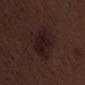Clinical impression: This lesion was catalogued during total-body skin photography and was not selected for biopsy. Image and clinical context: Measured at roughly 6 mm in maximum diameter. This is a white-light tile. Located on the left thigh. The subject is a male in their 70s. A close-up tile cropped from a whole-body skin photograph, about 15 mm across.